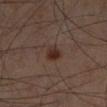The subject is a male aged 58 to 62. Imaged with cross-polarized lighting. Cropped from a whole-body photographic skin survey; the tile spans about 15 mm. Automated image analysis of the tile measured a footprint of about 4 mm², an eccentricity of roughly 0.75, and two-axis asymmetry of about 0.2. And it measured a mean CIELAB color near L≈25 a*≈15 b*≈20 and a lesion-to-skin contrast of about 9 (normalized; higher = more distinct). And it measured an automated nevus-likeness rating near 95 out of 100. The lesion's longest dimension is about 2.5 mm. The lesion is located on the leg.